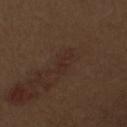workup: imaged on a skin check; not biopsied
lesion size: ≈3 mm
body site: the arm
patient: male, approximately 70 years of age
imaging modality: ~15 mm crop, total-body skin-cancer survey